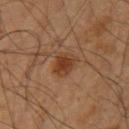workup: no biopsy performed (imaged during a skin exam) | anatomic site: the right upper arm | patient: male, roughly 60 years of age | acquisition: ~15 mm crop, total-body skin-cancer survey | illumination: cross-polarized illumination | lesion size: about 3 mm | TBP lesion metrics: a border-irregularity index near 2.5/10 and radial color variation of about 0.5.